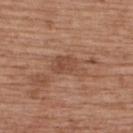size = ~4 mm (longest diameter) | imaging modality = 15 mm crop, total-body photography | body site = the back | patient = female, roughly 40 years of age | illumination = white-light.